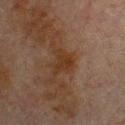<lesion>
  <biopsy_status>not biopsied; imaged during a skin examination</biopsy_status>
  <image>
    <source>total-body photography crop</source>
    <field_of_view_mm>15</field_of_view_mm>
  </image>
  <site>chest</site>
  <patient>
    <sex>male</sex>
    <age_approx>80</age_approx>
  </patient>
  <lighting>cross-polarized</lighting>
  <lesion_size>
    <long_diameter_mm_approx>3.0</long_diameter_mm_approx>
  </lesion_size>
</lesion>